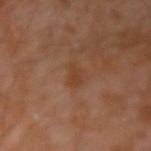notes: total-body-photography surveillance lesion; no biopsy | patient: male, approximately 30 years of age | anatomic site: the left forearm | imaging modality: 15 mm crop, total-body photography | automated lesion analysis: a lesion color around L≈39 a*≈21 b*≈31 in CIELAB, roughly 5 lightness units darker than nearby skin, and a normalized lesion–skin contrast near 6; border irregularity of about 4 on a 0–10 scale, a within-lesion color-variation index near 1/10, and radial color variation of about 0.5; a nevus-likeness score of about 0/100 | lighting: cross-polarized illumination | size: ~3 mm (longest diameter).